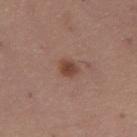– biopsy status · catalogued during a skin exam; not biopsied
– lighting · white-light
– image source · 15 mm crop, total-body photography
– anatomic site · the leg
– patient · female, roughly 60 years of age
– lesion diameter · ~2.5 mm (longest diameter)
– image-analysis metrics · a footprint of about 3.5 mm², a shape eccentricity near 0.7, and a symmetry-axis asymmetry near 0.25; a lesion color around L≈44 a*≈21 b*≈27 in CIELAB and a normalized border contrast of about 8.5; a nevus-likeness score of about 95/100 and a detector confidence of about 100 out of 100 that the crop contains a lesion